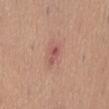{"biopsy_status": "not biopsied; imaged during a skin examination", "image": {"source": "total-body photography crop", "field_of_view_mm": 15}, "automated_metrics": {"area_mm2_approx": 3.0, "eccentricity": 0.9, "color_variation_0_10": 1.0, "peripheral_color_asymmetry": 0.0, "nevus_likeness_0_100": 0, "lesion_detection_confidence_0_100": 100}, "site": "abdomen", "patient": {"sex": "male", "age_approx": 55}, "lesion_size": {"long_diameter_mm_approx": 3.0}}